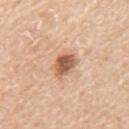notes: no biopsy performed (imaged during a skin exam) | automated metrics: a lesion color around L≈59 a*≈21 b*≈33 in CIELAB, a lesion–skin lightness drop of about 15, and a lesion-to-skin contrast of about 9.5 (normalized; higher = more distinct); a detector confidence of about 100 out of 100 that the crop contains a lesion | patient: male, roughly 70 years of age | body site: the mid back | imaging modality: ~15 mm crop, total-body skin-cancer survey.